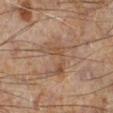notes: imaged on a skin check; not biopsied
diameter: about 5.5 mm
site: the left leg
acquisition: total-body-photography crop, ~15 mm field of view
subject: male, aged around 60
tile lighting: cross-polarized illumination